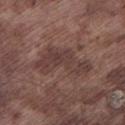The lesion was photographed on a routine skin check and not biopsied; there is no pathology result.
An algorithmic analysis of the crop reported a border-irregularity index near 7.5/10 and a within-lesion color-variation index near 3/10. The analysis additionally found a classifier nevus-likeness of about 0/100 and a detector confidence of about 65 out of 100 that the crop contains a lesion.
This is a white-light tile.
A male subject, about 75 years old.
Approximately 7 mm at its widest.
On the leg.
Cropped from a whole-body photographic skin survey; the tile spans about 15 mm.